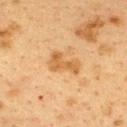notes = no biopsy performed (imaged during a skin exam) | patient = female, roughly 40 years of age | tile lighting = cross-polarized | image source = 15 mm crop, total-body photography | location = the upper back | lesion size = about 4 mm.